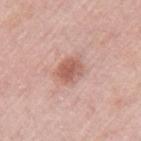workup: total-body-photography surveillance lesion; no biopsy | subject: female, approximately 65 years of age | image: 15 mm crop, total-body photography | site: the left upper arm.